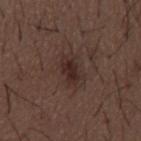Case summary:
- location: the mid back
- acquisition: total-body-photography crop, ~15 mm field of view
- illumination: white-light
- patient: male, aged around 50
- lesion diameter: ~3 mm (longest diameter)